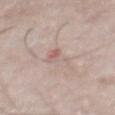Q: Was a biopsy performed?
A: imaged on a skin check; not biopsied
Q: What is the anatomic site?
A: the abdomen
Q: What are the patient's age and sex?
A: male, in their mid- to late 70s
Q: What is the imaging modality?
A: 15 mm crop, total-body photography